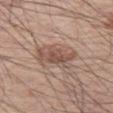Case summary:
– follow-up: imaged on a skin check; not biopsied
– subject: male, aged approximately 55
– diameter: about 4.5 mm
– automated lesion analysis: an average lesion color of about L≈52 a*≈18 b*≈25 (CIELAB) and a normalized lesion–skin contrast near 7; a border-irregularity index near 3.5/10, a within-lesion color-variation index near 4.5/10, and peripheral color asymmetry of about 1.5; a nevus-likeness score of about 55/100 and a lesion-detection confidence of about 100/100
– lighting: white-light illumination
– location: the left thigh
– acquisition: total-body-photography crop, ~15 mm field of view